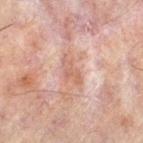* biopsy status · catalogued during a skin exam; not biopsied